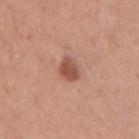Case summary:
• diameter — ≈2.5 mm
• tile lighting — white-light illumination
• subject — male, in their mid-50s
• image — total-body-photography crop, ~15 mm field of view
• anatomic site — the arm